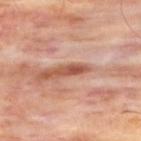Clinical impression: Part of a total-body skin-imaging series; this lesion was reviewed on a skin check and was not flagged for biopsy.